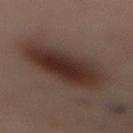  biopsy_status: not biopsied; imaged during a skin examination
  automated_metrics:
    area_mm2_approx: 34.0
    eccentricity: 0.9
    shape_asymmetry: 0.15
    cielab_L: 25
    cielab_a: 13
    cielab_b: 17
    vs_skin_darker_L: 10.0
    border_irregularity_0_10: 2.5
    peripheral_color_asymmetry: 1.5
  lesion_size:
    long_diameter_mm_approx: 10.5
  image:
    source: total-body photography crop
    field_of_view_mm: 15
  patient:
    sex: female
    age_approx: 55
  lighting: cross-polarized
  site: mid back